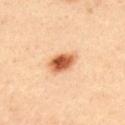notes=catalogued during a skin exam; not biopsied
tile lighting=cross-polarized
lesion size=≈4 mm
body site=the upper back
image-analysis metrics=a lesion area of about 7 mm² and a shape eccentricity near 0.75; a lesion-detection confidence of about 100/100
subject=male, aged approximately 35
imaging modality=15 mm crop, total-body photography